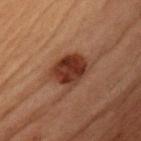Impression: The lesion was tiled from a total-body skin photograph and was not biopsied. Image and clinical context: A lesion tile, about 15 mm wide, cut from a 3D total-body photograph. About 4.5 mm across. The lesion is located on the chest. Automated image analysis of the tile measured a lesion area of about 12 mm² and a symmetry-axis asymmetry near 0.15. And it measured border irregularity of about 2 on a 0–10 scale and internal color variation of about 4 on a 0–10 scale. The analysis additionally found lesion-presence confidence of about 100/100. A male patient in their 50s.